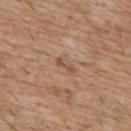  biopsy_status: not biopsied; imaged during a skin examination
  lighting: white-light
  automated_metrics:
    area_mm2_approx: 2.5
    eccentricity: 0.9
    shape_asymmetry: 0.3
    cielab_L: 52
    cielab_a: 19
    cielab_b: 31
    vs_skin_darker_L: 8.0
    nevus_likeness_0_100: 0
    lesion_detection_confidence_0_100: 100
  site: front of the torso
  patient:
    sex: male
    age_approx: 60
  image:
    source: total-body photography crop
    field_of_view_mm: 15